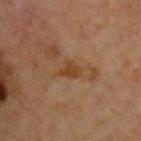Q: Was a biopsy performed?
A: no biopsy performed (imaged during a skin exam)
Q: Where on the body is the lesion?
A: the chest
Q: What lighting was used for the tile?
A: cross-polarized illumination
Q: What is the lesion's diameter?
A: ≈3.5 mm
Q: Who is the patient?
A: male, aged approximately 65
Q: How was this image acquired?
A: ~15 mm crop, total-body skin-cancer survey